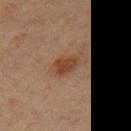Assessment:
Part of a total-body skin-imaging series; this lesion was reviewed on a skin check and was not flagged for biopsy.
Clinical summary:
The lesion is on the front of the torso. The total-body-photography lesion software estimated a nevus-likeness score of about 80/100 and lesion-presence confidence of about 100/100. A region of skin cropped from a whole-body photographic capture, roughly 15 mm wide. Approximately 3 mm at its widest. Captured under cross-polarized illumination. A male patient, roughly 65 years of age.